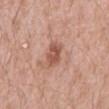workup — catalogued during a skin exam; not biopsied | subject — male, aged around 60 | size — about 3 mm | location — the abdomen | image source — 15 mm crop, total-body photography.